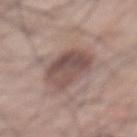Image and clinical context: The tile uses white-light illumination. The lesion's longest dimension is about 6 mm. The lesion-visualizer software estimated lesion-presence confidence of about 100/100. A close-up tile cropped from a whole-body skin photograph, about 15 mm across. A male patient, approximately 70 years of age. The lesion is on the back.